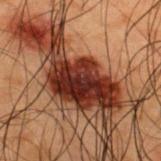Impression:
Recorded during total-body skin imaging; not selected for excision or biopsy.
Clinical summary:
On the upper back. The recorded lesion diameter is about 9 mm. A region of skin cropped from a whole-body photographic capture, roughly 15 mm wide. A male subject, in their 50s. Captured under cross-polarized illumination.